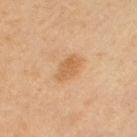Impression:
Imaged during a routine full-body skin examination; the lesion was not biopsied and no histopathology is available.